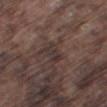Impression:
No biopsy was performed on this lesion — it was imaged during a full skin examination and was not determined to be concerning.
Acquisition and patient details:
From the right thigh. About 3 mm across. Automated tile analysis of the lesion measured a nevus-likeness score of about 0/100 and lesion-presence confidence of about 75/100. A male subject, in their mid-70s. Cropped from a total-body skin-imaging series; the visible field is about 15 mm. This is a white-light tile.